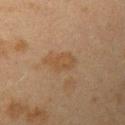acquisition=total-body-photography crop, ~15 mm field of view | TBP lesion metrics=a lesion area of about 6 mm² and two-axis asymmetry of about 0.3; roughly 5 lightness units darker than nearby skin; a border-irregularity rating of about 3/10, a within-lesion color-variation index near 1/10, and peripheral color asymmetry of about 0.5; a nevus-likeness score of about 0/100 and a lesion-detection confidence of about 100/100 | subject=female, in their 40s | tile lighting=cross-polarized | lesion diameter=about 4 mm | location=the arm.